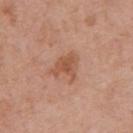Captured during whole-body skin photography for melanoma surveillance; the lesion was not biopsied. Captured under white-light illumination. Longest diameter approximately 4 mm. A male subject, aged 68 to 72. The lesion-visualizer software estimated an outline eccentricity of about 0.65 (0 = round, 1 = elongated). The software also gave a border-irregularity rating of about 4.5/10 and a peripheral color-asymmetry measure near 0.5. A 15 mm crop from a total-body photograph taken for skin-cancer surveillance. From the chest.